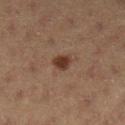Q: Was this lesion biopsied?
A: catalogued during a skin exam; not biopsied
Q: Lesion location?
A: the leg
Q: How was this image acquired?
A: ~15 mm crop, total-body skin-cancer survey
Q: Illumination type?
A: cross-polarized illumination
Q: Patient demographics?
A: female, aged around 40
Q: What is the lesion's diameter?
A: ~2.5 mm (longest diameter)